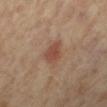notes: imaged on a skin check; not biopsied
image source: total-body-photography crop, ~15 mm field of view
patient: female, approximately 65 years of age
lighting: cross-polarized illumination
site: the leg
image-analysis metrics: a footprint of about 5.5 mm², an eccentricity of roughly 0.6, and a symmetry-axis asymmetry near 0.3; an average lesion color of about L≈45 a*≈20 b*≈27 (CIELAB) and a lesion–skin lightness drop of about 9; a border-irregularity index near 2.5/10, a within-lesion color-variation index near 2.5/10, and radial color variation of about 0.5
diameter: ~3 mm (longest diameter)